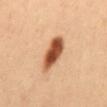Assessment: The lesion was photographed on a routine skin check and not biopsied; there is no pathology result.